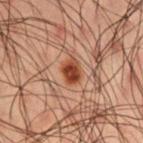Imaged during a routine full-body skin examination; the lesion was not biopsied and no histopathology is available. The recorded lesion diameter is about 2.5 mm. A 15 mm close-up extracted from a 3D total-body photography capture. Imaged with cross-polarized lighting. A male patient in their 50s. The lesion is on the right thigh. Automated image analysis of the tile measured an area of roughly 4.5 mm², an eccentricity of roughly 0.5, and two-axis asymmetry of about 0.15. The analysis additionally found a border-irregularity index near 1.5/10, internal color variation of about 3 on a 0–10 scale, and radial color variation of about 1.